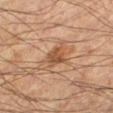{
  "biopsy_status": "not biopsied; imaged during a skin examination",
  "lesion_size": {
    "long_diameter_mm_approx": 3.0
  },
  "patient": {
    "sex": "male",
    "age_approx": 65
  },
  "image": {
    "source": "total-body photography crop",
    "field_of_view_mm": 15
  },
  "automated_metrics": {
    "area_mm2_approx": 5.5,
    "shape_asymmetry": 0.2,
    "cielab_L": 43,
    "cielab_a": 19,
    "cielab_b": 30,
    "vs_skin_darker_L": 9.0,
    "vs_skin_contrast_norm": 7.0
  },
  "site": "left lower leg",
  "lighting": "cross-polarized"
}